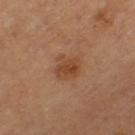notes — imaged on a skin check; not biopsied